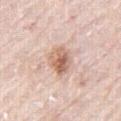follow-up: imaged on a skin check; not biopsied
illumination: white-light
anatomic site: the right thigh
lesion diameter: ~3.5 mm (longest diameter)
image: ~15 mm crop, total-body skin-cancer survey
subject: male, aged 78–82